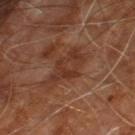follow-up: total-body-photography surveillance lesion; no biopsy
lesion diameter: about 5.5 mm
location: the right leg
subject: male, roughly 60 years of age
illumination: cross-polarized
image source: 15 mm crop, total-body photography
automated metrics: an area of roughly 11 mm², an eccentricity of roughly 0.85, and a shape-asymmetry score of about 0.35 (0 = symmetric); a lesion color around L≈33 a*≈21 b*≈27 in CIELAB and a lesion-to-skin contrast of about 6.5 (normalized; higher = more distinct); lesion-presence confidence of about 100/100